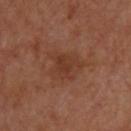The lesion was tiled from a total-body skin photograph and was not biopsied.
A patient in their mid-60s.
Imaged with cross-polarized lighting.
From the upper back.
A 15 mm crop from a total-body photograph taken for skin-cancer surveillance.